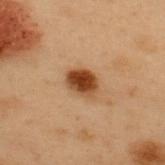{"biopsy_status": "not biopsied; imaged during a skin examination", "image": {"source": "total-body photography crop", "field_of_view_mm": 15}, "site": "upper back", "patient": {"sex": "male", "age_approx": 55}, "lighting": "cross-polarized", "lesion_size": {"long_diameter_mm_approx": 3.5}}